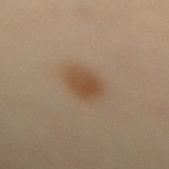Case summary:
• follow-up · catalogued during a skin exam; not biopsied
• lesion diameter · about 4 mm
• TBP lesion metrics · a nevus-likeness score of about 100/100
• patient · female, aged 58–62
• acquisition · ~15 mm crop, total-body skin-cancer survey
• body site · the back
• tile lighting · cross-polarized